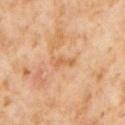Findings:
- follow-up · total-body-photography surveillance lesion; no biopsy
- body site · the chest
- image source · 15 mm crop, total-body photography
- subject · male, about 60 years old
- lesion diameter · ≈3 mm
- tile lighting · cross-polarized illumination